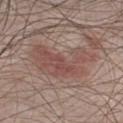biopsy status = catalogued during a skin exam; not biopsied | patient = male, about 30 years old | imaging modality = ~15 mm crop, total-body skin-cancer survey.